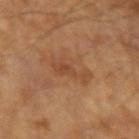Clinical impression:
The lesion was tiled from a total-body skin photograph and was not biopsied.